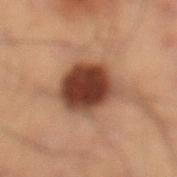Assessment: Captured during whole-body skin photography for melanoma surveillance; the lesion was not biopsied. Context: Measured at roughly 6.5 mm in maximum diameter. On the left lower leg. This is a cross-polarized tile. A male patient about 40 years old. A close-up tile cropped from a whole-body skin photograph, about 15 mm across.